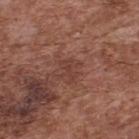<case>
<biopsy_status>not biopsied; imaged during a skin examination</biopsy_status>
<patient>
  <sex>male</sex>
  <age_approx>75</age_approx>
</patient>
<image>
  <source>total-body photography crop</source>
  <field_of_view_mm>15</field_of_view_mm>
</image>
<lesion_size>
  <long_diameter_mm_approx>2.5</long_diameter_mm_approx>
</lesion_size>
<automated_metrics>
  <area_mm2_approx>3.0</area_mm2_approx>
  <eccentricity>0.8</eccentricity>
  <shape_asymmetry>0.5</shape_asymmetry>
</automated_metrics>
<site>upper back</site>
</case>